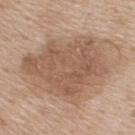A 15 mm close-up extracted from a 3D total-body photography capture.
Located on the upper back.
The subject is a male aged approximately 60.